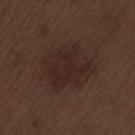workup: no biopsy performed (imaged during a skin exam)
illumination: white-light illumination
location: the left thigh
acquisition: total-body-photography crop, ~15 mm field of view
subject: male, about 70 years old
lesion diameter: about 6 mm